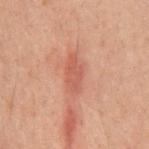notes: catalogued during a skin exam; not biopsied
body site: the chest
patient: male, aged around 60
image source: total-body-photography crop, ~15 mm field of view
lesion diameter: ≈4.5 mm